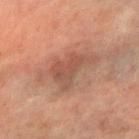Imaged with cross-polarized lighting.
From the right forearm.
A close-up tile cropped from a whole-body skin photograph, about 15 mm across.
The patient is a female aged 53–57.
The lesion-visualizer software estimated a mean CIELAB color near L≈45 a*≈20 b*≈26 and roughly 8 lightness units darker than nearby skin. And it measured border irregularity of about 6 on a 0–10 scale, a color-variation rating of about 4.5/10, and radial color variation of about 2.